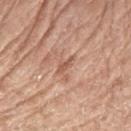Assessment:
No biopsy was performed on this lesion — it was imaged during a full skin examination and was not determined to be concerning.
Clinical summary:
The total-body-photography lesion software estimated a border-irregularity rating of about 3.5/10 and a peripheral color-asymmetry measure near 0. The software also gave a nevus-likeness score of about 0/100. The lesion is on the left forearm. This is a white-light tile. Cropped from a total-body skin-imaging series; the visible field is about 15 mm. A female patient aged 73–77.